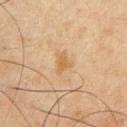size: about 3 mm | imaging modality: 15 mm crop, total-body photography | body site: the chest | subject: male, aged 43 to 47 | TBP lesion metrics: an average lesion color of about L≈50 a*≈15 b*≈33 (CIELAB), a lesion–skin lightness drop of about 6, and a lesion-to-skin contrast of about 6 (normalized; higher = more distinct); border irregularity of about 4 on a 0–10 scale, internal color variation of about 2 on a 0–10 scale, and radial color variation of about 0.5; an automated nevus-likeness rating near 0 out of 100 | lighting: cross-polarized illumination.